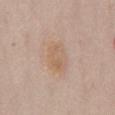Assessment:
This lesion was catalogued during total-body skin photography and was not selected for biopsy.
Image and clinical context:
The subject is a female aged 63–67. The lesion-visualizer software estimated radial color variation of about 1. Imaged with white-light lighting. A 15 mm close-up tile from a total-body photography series done for melanoma screening. On the abdomen. Longest diameter approximately 4 mm.